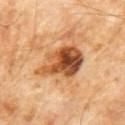An algorithmic analysis of the crop reported a border-irregularity index near 3.5/10, a color-variation rating of about 10/10, and radial color variation of about 4. Cropped from a whole-body photographic skin survey; the tile spans about 15 mm. Located on the chest. The tile uses cross-polarized illumination. The patient is a male aged 58 to 62. Longest diameter approximately 6 mm.